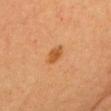follow-up: total-body-photography surveillance lesion; no biopsy
subject: female, aged 63 to 67
body site: the chest
imaging modality: ~15 mm tile from a whole-body skin photo
image-analysis metrics: a lesion area of about 3.5 mm², an eccentricity of roughly 0.8, and two-axis asymmetry of about 0.25; a mean CIELAB color near L≈47 a*≈23 b*≈39 and a lesion-to-skin contrast of about 8 (normalized; higher = more distinct); a border-irregularity rating of about 2.5/10
lesion size: ≈2.5 mm
tile lighting: cross-polarized illumination